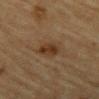Clinical impression:
No biopsy was performed on this lesion — it was imaged during a full skin examination and was not determined to be concerning.